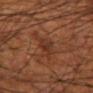Assessment: Captured during whole-body skin photography for melanoma surveillance; the lesion was not biopsied. Clinical summary: A close-up tile cropped from a whole-body skin photograph, about 15 mm across. The subject is a male aged approximately 55. This is a cross-polarized tile. The lesion is located on the arm. The lesion's longest dimension is about 4 mm.